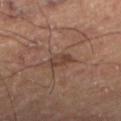Clinical impression: Part of a total-body skin-imaging series; this lesion was reviewed on a skin check and was not flagged for biopsy. Context: The lesion is located on the left lower leg. A close-up tile cropped from a whole-body skin photograph, about 15 mm across. The subject is a male approximately 55 years of age. About 3 mm across. This is a cross-polarized tile. Automated image analysis of the tile measured a classifier nevus-likeness of about 0/100 and a lesion-detection confidence of about 100/100.